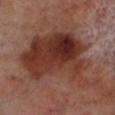Assessment:
Part of a total-body skin-imaging series; this lesion was reviewed on a skin check and was not flagged for biopsy.
Image and clinical context:
The lesion is located on the left lower leg. A male patient, aged around 70. A 15 mm close-up tile from a total-body photography series done for melanoma screening.